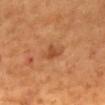Case summary:
• notes: catalogued during a skin exam; not biopsied
• image source: ~15 mm crop, total-body skin-cancer survey
• lesion size: about 3 mm
• patient: male, approximately 55 years of age
• anatomic site: the mid back
• lighting: cross-polarized